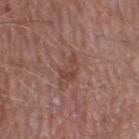The lesion was photographed on a routine skin check and not biopsied; there is no pathology result. Located on the right thigh. Measured at roughly 3 mm in maximum diameter. A roughly 15 mm field-of-view crop from a total-body skin photograph. The subject is a male roughly 55 years of age. The tile uses white-light illumination. Automated image analysis of the tile measured a lesion area of about 4 mm² and a symmetry-axis asymmetry near 0.55. The software also gave an average lesion color of about L≈44 a*≈22 b*≈24 (CIELAB), a lesion–skin lightness drop of about 7, and a normalized border contrast of about 6.